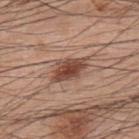| field | value |
|---|---|
| workup | no biopsy performed (imaged during a skin exam) |
| anatomic site | the upper back |
| image | total-body-photography crop, ~15 mm field of view |
| patient | male, aged approximately 60 |
| tile lighting | white-light |
| lesion diameter | about 4 mm |